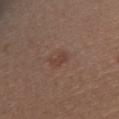<tbp_lesion>
  <biopsy_status>not biopsied; imaged during a skin examination</biopsy_status>
</tbp_lesion>